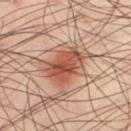A male subject, roughly 40 years of age.
A region of skin cropped from a whole-body photographic capture, roughly 15 mm wide.
On the leg.
The tile uses cross-polarized illumination.
The total-body-photography lesion software estimated a lesion area of about 17 mm², a shape eccentricity near 0.55, and a symmetry-axis asymmetry near 0.3. And it measured a border-irregularity rating of about 4.5/10, a color-variation rating of about 6.5/10, and a peripheral color-asymmetry measure near 2. The analysis additionally found a nevus-likeness score of about 100/100 and a lesion-detection confidence of about 100/100.